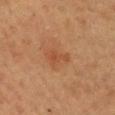The tile uses cross-polarized illumination. About 2.5 mm across. The lesion-visualizer software estimated a lesion area of about 3.5 mm², a shape eccentricity near 0.8, and a symmetry-axis asymmetry near 0.6. The lesion is located on the mid back. Cropped from a total-body skin-imaging series; the visible field is about 15 mm. A female patient in their 60s.